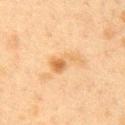Imaged during a routine full-body skin examination; the lesion was not biopsied and no histopathology is available. This is a cross-polarized tile. On the right upper arm. The recorded lesion diameter is about 4 mm. The subject is a female aged 38 to 42. Cropped from a total-body skin-imaging series; the visible field is about 15 mm.